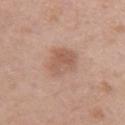Impression: Imaged during a routine full-body skin examination; the lesion was not biopsied and no histopathology is available. Acquisition and patient details: On the abdomen. A male subject, aged around 70. Measured at roughly 4 mm in maximum diameter. Cropped from a whole-body photographic skin survey; the tile spans about 15 mm.